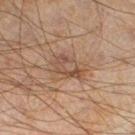Clinical impression: Recorded during total-body skin imaging; not selected for excision or biopsy. Clinical summary: About 3.5 mm across. A male patient aged 43 to 47. The lesion is on the left lower leg. A 15 mm close-up extracted from a 3D total-body photography capture. An algorithmic analysis of the crop reported a footprint of about 6.5 mm², an eccentricity of roughly 0.8, and a symmetry-axis asymmetry near 0.3. And it measured a nevus-likeness score of about 0/100 and lesion-presence confidence of about 100/100. Captured under cross-polarized illumination.